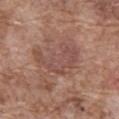Notes:
• notes: imaged on a skin check; not biopsied
• tile lighting: white-light illumination
• patient: male, approximately 75 years of age
• site: the abdomen
• image source: ~15 mm tile from a whole-body skin photo
• TBP lesion metrics: roughly 7 lightness units darker than nearby skin and a lesion-to-skin contrast of about 5.5 (normalized; higher = more distinct)